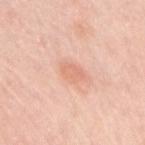<lesion>
  <biopsy_status>not biopsied; imaged during a skin examination</biopsy_status>
  <patient>
    <sex>female</sex>
    <age_approx>65</age_approx>
  </patient>
  <image>
    <source>total-body photography crop</source>
    <field_of_view_mm>15</field_of_view_mm>
  </image>
  <site>right upper arm</site>
</lesion>